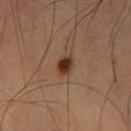Captured during whole-body skin photography for melanoma surveillance; the lesion was not biopsied. Approximately 2.5 mm at its widest. A male subject, aged approximately 60. Located on the left thigh. Cropped from a whole-body photographic skin survey; the tile spans about 15 mm. The total-body-photography lesion software estimated two-axis asymmetry of about 0.25. It also reported a mean CIELAB color near L≈34 a*≈20 b*≈28, roughly 13 lightness units darker than nearby skin, and a normalized border contrast of about 12. And it measured border irregularity of about 2 on a 0–10 scale, a color-variation rating of about 4/10, and peripheral color asymmetry of about 1.